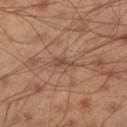Clinical impression: Part of a total-body skin-imaging series; this lesion was reviewed on a skin check and was not flagged for biopsy. Background: About 2.5 mm across. This is a cross-polarized tile. The subject is a male aged 43–47. An algorithmic analysis of the crop reported a border-irregularity index near 3.5/10 and a peripheral color-asymmetry measure near 0. It also reported lesion-presence confidence of about 85/100. The lesion is located on the left lower leg. A roughly 15 mm field-of-view crop from a total-body skin photograph.